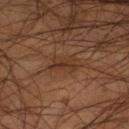This lesion was catalogued during total-body skin photography and was not selected for biopsy. A lesion tile, about 15 mm wide, cut from a 3D total-body photograph. A male subject, aged around 55. Approximately 3.5 mm at its widest. This is a cross-polarized tile. Automated tile analysis of the lesion measured an area of roughly 4.5 mm², an eccentricity of roughly 0.85, and a symmetry-axis asymmetry near 0.35. The analysis additionally found an automated nevus-likeness rating near 0 out of 100 and lesion-presence confidence of about 90/100. From the right lower leg.